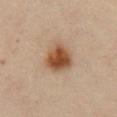The lesion was tiled from a total-body skin photograph and was not biopsied. The lesion is on the chest. The patient is a female in their 60s. A roughly 15 mm field-of-view crop from a total-body skin photograph.